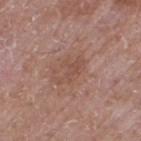Part of a total-body skin-imaging series; this lesion was reviewed on a skin check and was not flagged for biopsy.
Imaged with white-light lighting.
The lesion is on the right thigh.
A male patient about 60 years old.
A region of skin cropped from a whole-body photographic capture, roughly 15 mm wide.